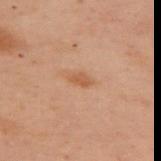The lesion was photographed on a routine skin check and not biopsied; there is no pathology result.
From the upper back.
The subject is a female approximately 55 years of age.
The lesion's longest dimension is about 2.5 mm.
A region of skin cropped from a whole-body photographic capture, roughly 15 mm wide.
The total-body-photography lesion software estimated an area of roughly 2.5 mm², an eccentricity of roughly 0.85, and a symmetry-axis asymmetry near 0.2. It also reported a peripheral color-asymmetry measure near 0.5.